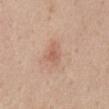Impression: The lesion was photographed on a routine skin check and not biopsied; there is no pathology result. Clinical summary: This is a white-light tile. The lesion is on the abdomen. About 3 mm across. A region of skin cropped from a whole-body photographic capture, roughly 15 mm wide. The subject is a male roughly 60 years of age.